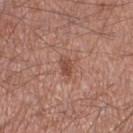lighting: white-light
patient:
  sex: male
  age_approx: 55
image:
  source: total-body photography crop
  field_of_view_mm: 15
site: leg